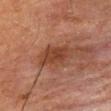Impression:
Recorded during total-body skin imaging; not selected for excision or biopsy.
Image and clinical context:
A male subject aged approximately 65. Approximately 4 mm at its widest. On the mid back. Cropped from a whole-body photographic skin survey; the tile spans about 15 mm.